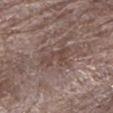Q: Was this lesion biopsied?
A: no biopsy performed (imaged during a skin exam)
Q: What is the imaging modality?
A: total-body-photography crop, ~15 mm field of view
Q: What lighting was used for the tile?
A: white-light illumination
Q: What did automated image analysis measure?
A: an area of roughly 5.5 mm², an outline eccentricity of about 0.9 (0 = round, 1 = elongated), and two-axis asymmetry of about 0.45; roughly 7 lightness units darker than nearby skin and a lesion-to-skin contrast of about 6 (normalized; higher = more distinct); border irregularity of about 6 on a 0–10 scale and peripheral color asymmetry of about 0.5
Q: Lesion location?
A: the left lower leg
Q: Who is the patient?
A: male, approximately 70 years of age
Q: How large is the lesion?
A: ≈4 mm